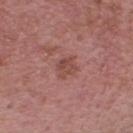notes — no biopsy performed (imaged during a skin exam) | subject — male, aged 48–52 | diameter — ≈3 mm | lighting — white-light | imaging modality — 15 mm crop, total-body photography | body site — the head or neck.